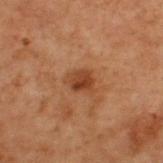Measured at roughly 3 mm in maximum diameter. Cropped from a whole-body photographic skin survey; the tile spans about 15 mm. The tile uses cross-polarized illumination. From the upper back. A male patient aged around 70. An algorithmic analysis of the crop reported a lesion color around L≈32 a*≈20 b*≈28 in CIELAB, roughly 8 lightness units darker than nearby skin, and a normalized lesion–skin contrast near 8. The software also gave a border-irregularity index near 2.5/10, a within-lesion color-variation index near 3.5/10, and a peripheral color-asymmetry measure near 1. The analysis additionally found a detector confidence of about 100 out of 100 that the crop contains a lesion.